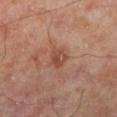{
  "biopsy_status": "not biopsied; imaged during a skin examination",
  "lighting": "cross-polarized",
  "patient": {
    "sex": "male",
    "age_approx": 50
  },
  "site": "left lower leg",
  "lesion_size": {
    "long_diameter_mm_approx": 3.0
  },
  "automated_metrics": {
    "cielab_L": 47,
    "cielab_a": 24,
    "cielab_b": 28,
    "vs_skin_darker_L": 8.0,
    "vs_skin_contrast_norm": 6.5,
    "border_irregularity_0_10": 2.5,
    "color_variation_0_10": 3.5,
    "peripheral_color_asymmetry": 1.5,
    "nevus_likeness_0_100": 5
  },
  "image": {
    "source": "total-body photography crop",
    "field_of_view_mm": 15
  }
}